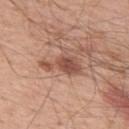Q: Was this lesion biopsied?
A: no biopsy performed (imaged during a skin exam)
Q: Illumination type?
A: white-light
Q: What is the anatomic site?
A: the upper back
Q: How was this image acquired?
A: total-body-photography crop, ~15 mm field of view
Q: Who is the patient?
A: male, aged around 55
Q: Lesion size?
A: ≈5 mm